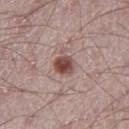biopsy_status: not biopsied; imaged during a skin examination
image:
  source: total-body photography crop
  field_of_view_mm: 15
site: left thigh
patient:
  sex: male
  age_approx: 20